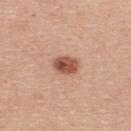biopsy_status: not biopsied; imaged during a skin examination
site: upper back
patient:
  sex: female
  age_approx: 40
image:
  source: total-body photography crop
  field_of_view_mm: 15